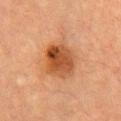Case summary:
• biopsy status · catalogued during a skin exam; not biopsied
• anatomic site · the chest
• lesion diameter · ≈4 mm
• patient · female, aged approximately 60
• automated metrics · a border-irregularity index near 1.5/10, a color-variation rating of about 7/10, and radial color variation of about 2.5
• image · 15 mm crop, total-body photography